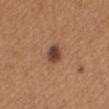| feature | finding |
|---|---|
| notes | imaged on a skin check; not biopsied |
| acquisition | 15 mm crop, total-body photography |
| subject | female, aged around 35 |
| illumination | white-light |
| site | the mid back |